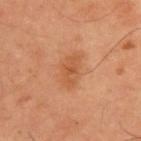Case summary:
– biopsy status · total-body-photography surveillance lesion; no biopsy
– tile lighting · cross-polarized
– subject · male, in their mid-50s
– image source · total-body-photography crop, ~15 mm field of view
– image-analysis metrics · a lesion color around L≈54 a*≈25 b*≈37 in CIELAB, about 7 CIELAB-L* units darker than the surrounding skin, and a normalized lesion–skin contrast near 5.5; a border-irregularity index near 3/10, internal color variation of about 2.5 on a 0–10 scale, and a peripheral color-asymmetry measure near 0.5
– location · the upper back